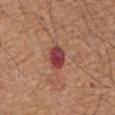workup: imaged on a skin check; not biopsied
diameter: about 3 mm
patient: male, aged 63–67
acquisition: 15 mm crop, total-body photography
lighting: white-light
TBP lesion metrics: a footprint of about 5 mm², an outline eccentricity of about 0.75 (0 = round, 1 = elongated), and a symmetry-axis asymmetry near 0.15; a mean CIELAB color near L≈42 a*≈32 b*≈23 and a normalized lesion–skin contrast near 12.5; a border-irregularity index near 1.5/10 and a within-lesion color-variation index near 7/10; a lesion-detection confidence of about 100/100
location: the abdomen